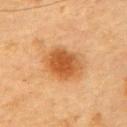On the front of the torso.
The subject is a male aged around 85 or older.
An algorithmic analysis of the crop reported a footprint of about 14 mm², a shape eccentricity near 0.7, and two-axis asymmetry of about 0.15. The analysis additionally found a mean CIELAB color near L≈48 a*≈24 b*≈39 and a normalized border contrast of about 9. And it measured a color-variation rating of about 4.5/10 and peripheral color asymmetry of about 1.
Approximately 5 mm at its widest.
The tile uses cross-polarized illumination.
This image is a 15 mm lesion crop taken from a total-body photograph.